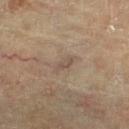The lesion was photographed on a routine skin check and not biopsied; there is no pathology result. From the right lower leg. A 15 mm crop from a total-body photograph taken for skin-cancer surveillance. The subject is a female aged approximately 80. This is a cross-polarized tile.